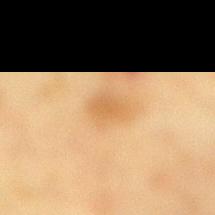Clinical impression: The lesion was photographed on a routine skin check and not biopsied; there is no pathology result. Context: The recorded lesion diameter is about 2.5 mm. The lesion is located on the right lower leg. A 15 mm close-up tile from a total-body photography series done for melanoma screening. A female subject, about 55 years old. This is a cross-polarized tile.